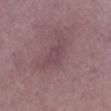notes: no biopsy performed (imaged during a skin exam) | lesion diameter: about 3 mm | TBP lesion metrics: an area of roughly 4.5 mm², an outline eccentricity of about 0.75 (0 = round, 1 = elongated), and two-axis asymmetry of about 0.3; a mean CIELAB color near L≈44 a*≈20 b*≈13 and a lesion-to-skin contrast of about 4.5 (normalized; higher = more distinct); a border-irregularity rating of about 3/10, internal color variation of about 1.5 on a 0–10 scale, and peripheral color asymmetry of about 0.5 | acquisition: 15 mm crop, total-body photography | lighting: white-light | location: the right lower leg | patient: female, about 60 years old.